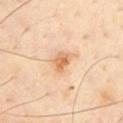No biopsy was performed on this lesion — it was imaged during a full skin examination and was not determined to be concerning. The total-body-photography lesion software estimated a symmetry-axis asymmetry near 0.3. And it measured a normalized border contrast of about 7. The analysis additionally found a border-irregularity rating of about 2.5/10, a color-variation rating of about 4/10, and peripheral color asymmetry of about 1.5. The software also gave a lesion-detection confidence of about 100/100. The subject is a male about 45 years old. Measured at roughly 3 mm in maximum diameter. Cropped from a whole-body photographic skin survey; the tile spans about 15 mm. From the front of the torso. Captured under cross-polarized illumination.